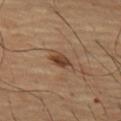  biopsy_status: not biopsied; imaged during a skin examination
  patient:
    sex: male
    age_approx: 65
  site: left thigh
  image:
    source: total-body photography crop
    field_of_view_mm: 15
  automated_metrics:
    area_mm2_approx: 3.5
    shape_asymmetry: 0.2